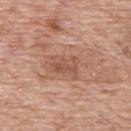No biopsy was performed on this lesion — it was imaged during a full skin examination and was not determined to be concerning. Captured under white-light illumination. The lesion is located on the upper back. A male patient aged 68 to 72. A close-up tile cropped from a whole-body skin photograph, about 15 mm across.